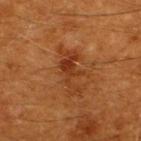Q: Is there a histopathology result?
A: imaged on a skin check; not biopsied
Q: Automated lesion metrics?
A: an area of roughly 8.5 mm², a shape eccentricity near 0.9, and a symmetry-axis asymmetry near 0.55; a nevus-likeness score of about 15/100 and a detector confidence of about 100 out of 100 that the crop contains a lesion
Q: What lighting was used for the tile?
A: cross-polarized
Q: Who is the patient?
A: male, roughly 60 years of age
Q: What kind of image is this?
A: ~15 mm tile from a whole-body skin photo
Q: Lesion location?
A: the upper back